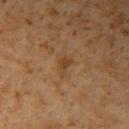* notes — catalogued during a skin exam; not biopsied
* size — ~2.5 mm (longest diameter)
* anatomic site — the right upper arm
* patient — male, roughly 60 years of age
* imaging modality — total-body-photography crop, ~15 mm field of view